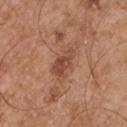image-analysis metrics: a lesion area of about 6 mm², a shape eccentricity near 0.85, and a shape-asymmetry score of about 0.35 (0 = symmetric); about 10 CIELAB-L* units darker than the surrounding skin and a normalized border contrast of about 7.5; lesion-presence confidence of about 100/100
anatomic site: the right upper arm
image source: ~15 mm crop, total-body skin-cancer survey
tile lighting: white-light illumination
subject: male, roughly 55 years of age